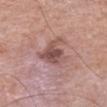Automated image analysis of the tile measured a classifier nevus-likeness of about 5/100 and lesion-presence confidence of about 100/100.
A male subject about 70 years old.
The tile uses white-light illumination.
Approximately 4.5 mm at its widest.
A region of skin cropped from a whole-body photographic capture, roughly 15 mm wide.
From the abdomen.